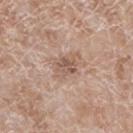Background: From the leg. A lesion tile, about 15 mm wide, cut from a 3D total-body photograph. This is a white-light tile. A male subject, roughly 60 years of age. Automated tile analysis of the lesion measured a footprint of about 5 mm². And it measured about 9 CIELAB-L* units darker than the surrounding skin. It also reported a nevus-likeness score of about 0/100.